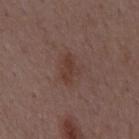Findings:
– workup: total-body-photography surveillance lesion; no biopsy
– subject: male, approximately 50 years of age
– tile lighting: white-light
– body site: the back
– diameter: ~3 mm (longest diameter)
– image source: ~15 mm tile from a whole-body skin photo